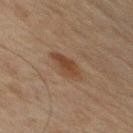This lesion was catalogued during total-body skin photography and was not selected for biopsy. A close-up tile cropped from a whole-body skin photograph, about 15 mm across. About 4 mm across. The lesion-visualizer software estimated a shape-asymmetry score of about 0.2 (0 = symmetric). The analysis additionally found lesion-presence confidence of about 100/100. Imaged with cross-polarized lighting. The subject is a male aged around 65. The lesion is located on the mid back.